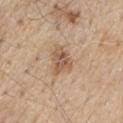The lesion was photographed on a routine skin check and not biopsied; there is no pathology result.
An algorithmic analysis of the crop reported an average lesion color of about L≈56 a*≈17 b*≈32 (CIELAB), a lesion–skin lightness drop of about 11, and a lesion-to-skin contrast of about 7 (normalized; higher = more distinct). And it measured a classifier nevus-likeness of about 25/100.
A region of skin cropped from a whole-body photographic capture, roughly 15 mm wide.
Longest diameter approximately 3.5 mm.
Imaged with white-light lighting.
A male patient about 70 years old.
Located on the chest.